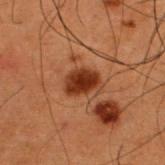follow-up = total-body-photography surveillance lesion; no biopsy
patient = male, aged approximately 50
acquisition = total-body-photography crop, ~15 mm field of view
body site = the upper back
size = about 3.5 mm